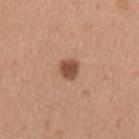{
  "automated_metrics": {
    "cielab_L": 50,
    "cielab_a": 24,
    "cielab_b": 30,
    "vs_skin_darker_L": 14.0,
    "vs_skin_contrast_norm": 9.5,
    "nevus_likeness_0_100": 95
  },
  "lighting": "white-light",
  "site": "left upper arm",
  "lesion_size": {
    "long_diameter_mm_approx": 2.5
  },
  "image": {
    "source": "total-body photography crop",
    "field_of_view_mm": 15
  },
  "patient": {
    "sex": "female",
    "age_approx": 40
  }
}